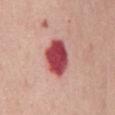notes: imaged on a skin check; not biopsied
acquisition: ~15 mm crop, total-body skin-cancer survey
body site: the abdomen
subject: female, about 65 years old
automated metrics: a lesion color around L≈48 a*≈37 b*≈23 in CIELAB, about 21 CIELAB-L* units darker than the surrounding skin, and a lesion-to-skin contrast of about 13.5 (normalized; higher = more distinct); an automated nevus-likeness rating near 0 out of 100